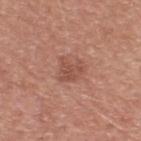• notes: total-body-photography surveillance lesion; no biopsy
• anatomic site: the upper back
• lesion size: ≈2.5 mm
• automated lesion analysis: an eccentricity of roughly 0.45 and a symmetry-axis asymmetry near 0.4; a lesion color around L≈51 a*≈24 b*≈28 in CIELAB, about 8 CIELAB-L* units darker than the surrounding skin, and a normalized border contrast of about 6; a border-irregularity index near 4.5/10
• image: ~15 mm crop, total-body skin-cancer survey
• patient: male, roughly 50 years of age
• tile lighting: white-light illumination